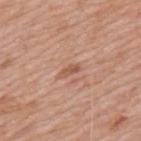Findings:
• notes · imaged on a skin check; not biopsied
• subject · male, aged 58 to 62
• diameter · ~2.5 mm (longest diameter)
• image source · 15 mm crop, total-body photography
• site · the mid back
• image-analysis metrics · a border-irregularity rating of about 2.5/10 and a within-lesion color-variation index near 0/10
• tile lighting · white-light illumination